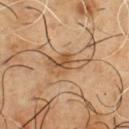biopsy_status: not biopsied; imaged during a skin examination
patient:
  sex: male
  age_approx: 50
automated_metrics:
  cielab_L: 54
  cielab_a: 19
  cielab_b: 36
  vs_skin_darker_L: 9.0
  vs_skin_contrast_norm: 7.0
  color_variation_0_10: 6.5
  peripheral_color_asymmetry: 2.5
  nevus_likeness_0_100: 5
  lesion_detection_confidence_0_100: 100
image:
  source: total-body photography crop
  field_of_view_mm: 15
lesion_size:
  long_diameter_mm_approx: 5.0
lighting: cross-polarized
site: front of the torso